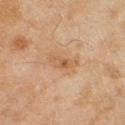Part of a total-body skin-imaging series; this lesion was reviewed on a skin check and was not flagged for biopsy. From the left lower leg. A region of skin cropped from a whole-body photographic capture, roughly 15 mm wide. A female patient, approximately 60 years of age.